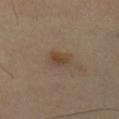workup: no biopsy performed (imaged during a skin exam) | automated lesion analysis: a lesion color around L≈43 a*≈15 b*≈28 in CIELAB and roughly 7 lightness units darker than nearby skin; a nevus-likeness score of about 40/100 and a detector confidence of about 100 out of 100 that the crop contains a lesion | patient: male, aged 53 to 57 | image: ~15 mm crop, total-body skin-cancer survey | site: the chest.